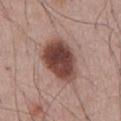Imaged during a routine full-body skin examination; the lesion was not biopsied and no histopathology is available.
This is a white-light tile.
This image is a 15 mm lesion crop taken from a total-body photograph.
A male patient, aged 53 to 57.
Longest diameter approximately 5.5 mm.
The lesion is on the abdomen.